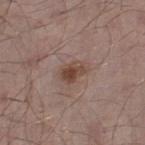notes=total-body-photography surveillance lesion; no biopsy
patient=male, aged 53–57
location=the right lower leg
acquisition=~15 mm crop, total-body skin-cancer survey